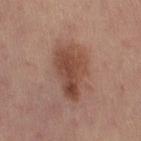Assessment: No biopsy was performed on this lesion — it was imaged during a full skin examination and was not determined to be concerning. Acquisition and patient details: A 15 mm close-up extracted from a 3D total-body photography capture. Captured under white-light illumination. From the right thigh. Longest diameter approximately 6 mm. A female patient approximately 25 years of age. The total-body-photography lesion software estimated an average lesion color of about L≈47 a*≈21 b*≈28 (CIELAB). And it measured a border-irregularity rating of about 4/10, internal color variation of about 5.5 on a 0–10 scale, and peripheral color asymmetry of about 2. It also reported an automated nevus-likeness rating near 55 out of 100 and a lesion-detection confidence of about 100/100.